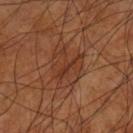Q: Was this lesion biopsied?
A: no biopsy performed (imaged during a skin exam)
Q: What is the anatomic site?
A: the left lower leg
Q: What are the patient's age and sex?
A: male, aged 58 to 62
Q: What kind of image is this?
A: ~15 mm crop, total-body skin-cancer survey